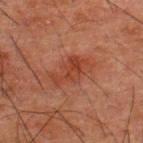<tbp_lesion>
  <biopsy_status>not biopsied; imaged during a skin examination</biopsy_status>
  <automated_metrics>
    <area_mm2_approx>9.5</area_mm2_approx>
    <eccentricity>0.85</eccentricity>
    <vs_skin_darker_L>6.0</vs_skin_darker_L>
    <vs_skin_contrast_norm>6.5</vs_skin_contrast_norm>
    <border_irregularity_0_10>4.5</border_irregularity_0_10>
    <color_variation_0_10>3.0</color_variation_0_10>
    <peripheral_color_asymmetry>1.0</peripheral_color_asymmetry>
  </automated_metrics>
  <lesion_size>
    <long_diameter_mm_approx>5.0</long_diameter_mm_approx>
  </lesion_size>
  <patient>
    <sex>male</sex>
    <age_approx>50</age_approx>
  </patient>
  <lighting>cross-polarized</lighting>
  <image>
    <source>total-body photography crop</source>
    <field_of_view_mm>15</field_of_view_mm>
  </image>
  <site>upper back</site>
</tbp_lesion>